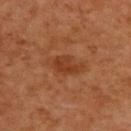notes = catalogued during a skin exam; not biopsied
lesion size = ~3.5 mm (longest diameter)
site = the upper back
subject = male, about 60 years old
tile lighting = cross-polarized
imaging modality = ~15 mm crop, total-body skin-cancer survey
image-analysis metrics = a lesion area of about 5 mm² and a shape eccentricity near 0.8; a border-irregularity rating of about 3/10, a within-lesion color-variation index near 2.5/10, and radial color variation of about 1